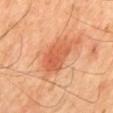Captured during whole-body skin photography for melanoma surveillance; the lesion was not biopsied. From the mid back. A male patient approximately 45 years of age. This is a cross-polarized tile. A 15 mm crop from a total-body photograph taken for skin-cancer surveillance.